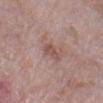Q: Was a biopsy performed?
A: total-body-photography surveillance lesion; no biopsy
Q: What are the patient's age and sex?
A: female, in their 70s
Q: What is the anatomic site?
A: the left lower leg
Q: What is the imaging modality?
A: ~15 mm crop, total-body skin-cancer survey
Q: How was the tile lit?
A: white-light illumination
Q: Lesion size?
A: about 2.5 mm